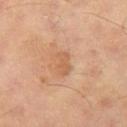Part of a total-body skin-imaging series; this lesion was reviewed on a skin check and was not flagged for biopsy. A male subject, about 65 years old. This is a cross-polarized tile. About 3 mm across. Located on the left thigh. A lesion tile, about 15 mm wide, cut from a 3D total-body photograph. The total-body-photography lesion software estimated an area of roughly 4 mm² and an eccentricity of roughly 0.85. The analysis additionally found roughly 7 lightness units darker than nearby skin. The software also gave a border-irregularity rating of about 3/10.